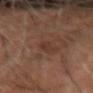A 15 mm crop from a total-body photograph taken for skin-cancer surveillance.
This is a cross-polarized tile.
A male patient, in their 70s.
Located on the right forearm.